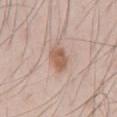Recorded during total-body skin imaging; not selected for excision or biopsy. On the abdomen. A 15 mm close-up tile from a total-body photography series done for melanoma screening. A male patient in their mid-40s.